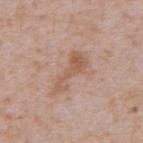Notes:
- workup — catalogued during a skin exam; not biopsied
- anatomic site — the abdomen
- subject — male, aged 63–67
- lesion diameter — ≈5.5 mm
- image source — 15 mm crop, total-body photography
- automated metrics — a footprint of about 8 mm², an outline eccentricity of about 0.95 (0 = round, 1 = elongated), and a symmetry-axis asymmetry near 0.45; a mean CIELAB color near L≈57 a*≈20 b*≈29, a lesion–skin lightness drop of about 8, and a normalized border contrast of about 6.5; border irregularity of about 6 on a 0–10 scale and peripheral color asymmetry of about 0.5; a nevus-likeness score of about 0/100 and a lesion-detection confidence of about 100/100
- lighting — white-light